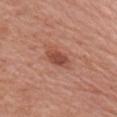This lesion was catalogued during total-body skin photography and was not selected for biopsy. Cropped from a whole-body photographic skin survey; the tile spans about 15 mm. Located on the right upper arm. A male subject, aged 53–57. This is a white-light tile. An algorithmic analysis of the crop reported an area of roughly 5.5 mm², an eccentricity of roughly 0.75, and a symmetry-axis asymmetry near 0.15.